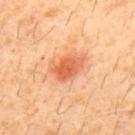Q: Was a biopsy performed?
A: catalogued during a skin exam; not biopsied
Q: What is the lesion's diameter?
A: ≈4 mm
Q: What lighting was used for the tile?
A: cross-polarized illumination
Q: What is the anatomic site?
A: the upper back
Q: What is the imaging modality?
A: ~15 mm crop, total-body skin-cancer survey
Q: Patient demographics?
A: male, aged 28 to 32
Q: What did automated image analysis measure?
A: internal color variation of about 5 on a 0–10 scale and peripheral color asymmetry of about 1.5